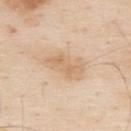biopsy_status: not biopsied; imaged during a skin examination
patient:
  sex: male
  age_approx: 80
site: upper back
lighting: white-light
lesion_size:
  long_diameter_mm_approx: 5.5
image:
  source: total-body photography crop
  field_of_view_mm: 15